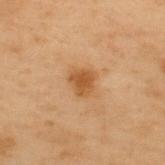Assessment:
Imaged during a routine full-body skin examination; the lesion was not biopsied and no histopathology is available.
Context:
The subject is a male aged approximately 55. Approximately 2.5 mm at its widest. An algorithmic analysis of the crop reported an outline eccentricity of about 0.45 (0 = round, 1 = elongated) and a symmetry-axis asymmetry near 0.3. The software also gave a mean CIELAB color near L≈43 a*≈19 b*≈35 and a lesion-to-skin contrast of about 8 (normalized; higher = more distinct). The tile uses cross-polarized illumination. On the upper back. Cropped from a total-body skin-imaging series; the visible field is about 15 mm.